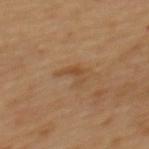Findings:
* notes: total-body-photography surveillance lesion; no biopsy
* image source: ~15 mm tile from a whole-body skin photo
* lesion diameter: ≈3 mm
* subject: male, roughly 60 years of age
* tile lighting: cross-polarized
* body site: the back
* automated metrics: an area of roughly 3.5 mm², an outline eccentricity of about 0.8 (0 = round, 1 = elongated), and a symmetry-axis asymmetry near 0.65; a lesion color around L≈46 a*≈18 b*≈33 in CIELAB and roughly 7 lightness units darker than nearby skin; a border-irregularity rating of about 7/10, a within-lesion color-variation index near 0/10, and radial color variation of about 0